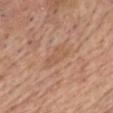follow-up: total-body-photography surveillance lesion; no biopsy
subject: male, roughly 60 years of age
lighting: white-light
anatomic site: the head or neck
image-analysis metrics: a footprint of about 5.5 mm², a shape eccentricity near 0.85, and a symmetry-axis asymmetry near 0.35; a lesion color around L≈56 a*≈20 b*≈32 in CIELAB and a lesion-to-skin contrast of about 4.5 (normalized; higher = more distinct); a detector confidence of about 100 out of 100 that the crop contains a lesion
lesion diameter: ~3.5 mm (longest diameter)
imaging modality: total-body-photography crop, ~15 mm field of view